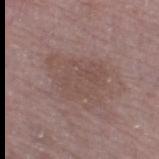diameter: ~6.5 mm (longest diameter); anatomic site: the right thigh; subject: female, in their mid- to late 60s; lighting: white-light illumination; TBP lesion metrics: an eccentricity of roughly 0.7 and two-axis asymmetry of about 0.4; imaging modality: 15 mm crop, total-body photography.